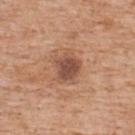Assessment:
Captured during whole-body skin photography for melanoma surveillance; the lesion was not biopsied.
Acquisition and patient details:
An algorithmic analysis of the crop reported an average lesion color of about L≈50 a*≈22 b*≈29 (CIELAB) and a lesion-to-skin contrast of about 8.5 (normalized; higher = more distinct). The analysis additionally found a within-lesion color-variation index near 3.5/10 and radial color variation of about 1. The lesion's longest dimension is about 3 mm. The tile uses white-light illumination. The lesion is located on the upper back. A 15 mm crop from a total-body photograph taken for skin-cancer surveillance. A male patient roughly 60 years of age.